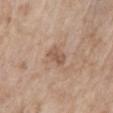  biopsy_status: not biopsied; imaged during a skin examination
  lighting: white-light
  image:
    source: total-body photography crop
    field_of_view_mm: 15
  lesion_size:
    long_diameter_mm_approx: 3.0
  patient:
    sex: female
    age_approx: 75
  site: mid back